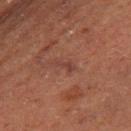Case summary:
* workup: total-body-photography surveillance lesion; no biopsy
* imaging modality: ~15 mm tile from a whole-body skin photo
* diameter: ~2.5 mm (longest diameter)
* illumination: cross-polarized illumination
* automated metrics: a footprint of about 2 mm² and a symmetry-axis asymmetry near 0.45; an average lesion color of about L≈31 a*≈19 b*≈20 (CIELAB), about 5 CIELAB-L* units darker than the surrounding skin, and a normalized lesion–skin contrast near 6; border irregularity of about 5 on a 0–10 scale, a within-lesion color-variation index near 0/10, and a peripheral color-asymmetry measure near 0; a classifier nevus-likeness of about 0/100 and a lesion-detection confidence of about 95/100
* patient: male, aged 73 to 77
* location: the right thigh